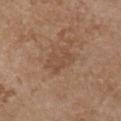Notes:
* notes · total-body-photography surveillance lesion; no biopsy
* acquisition · ~15 mm tile from a whole-body skin photo
* patient · female, in their mid- to late 60s
* anatomic site · the front of the torso
* TBP lesion metrics · a lesion color around L≈48 a*≈19 b*≈30 in CIELAB and a lesion-to-skin contrast of about 4.5 (normalized; higher = more distinct)
* illumination · white-light
* lesion size · ~3.5 mm (longest diameter)